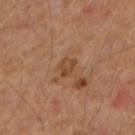No biopsy was performed on this lesion — it was imaged during a full skin examination and was not determined to be concerning. A female subject, roughly 60 years of age. A close-up tile cropped from a whole-body skin photograph, about 15 mm across. Imaged with cross-polarized lighting. The lesion is on the right forearm.